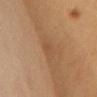Part of a total-body skin-imaging series; this lesion was reviewed on a skin check and was not flagged for biopsy. This is a cross-polarized tile. The lesion is on the chest. The subject is a female about 35 years old. A 15 mm close-up extracted from a 3D total-body photography capture.